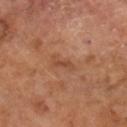Clinical impression:
Recorded during total-body skin imaging; not selected for excision or biopsy.
Clinical summary:
The total-body-photography lesion software estimated a lesion color around L≈45 a*≈23 b*≈32 in CIELAB, about 7 CIELAB-L* units darker than the surrounding skin, and a lesion-to-skin contrast of about 5.5 (normalized; higher = more distinct). The subject is a male in their mid- to late 60s. A region of skin cropped from a whole-body photographic capture, roughly 15 mm wide. Approximately 2.5 mm at its widest.